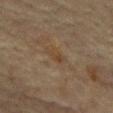Clinical impression: Recorded during total-body skin imaging; not selected for excision or biopsy. Context: From the mid back. An algorithmic analysis of the crop reported an area of roughly 3.5 mm², a shape eccentricity near 0.8, and a shape-asymmetry score of about 0.35 (0 = symmetric). It also reported a lesion-to-skin contrast of about 5.5 (normalized; higher = more distinct). And it measured a border-irregularity index near 3.5/10 and a color-variation rating of about 3/10. It also reported a nevus-likeness score of about 0/100. The recorded lesion diameter is about 2.5 mm. A 15 mm crop from a total-body photograph taken for skin-cancer surveillance. The patient is a male roughly 65 years of age.